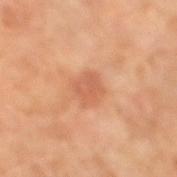biopsy status: imaged on a skin check; not biopsied
site: the right lower leg
patient: female, roughly 70 years of age
lighting: cross-polarized illumination
image: ~15 mm crop, total-body skin-cancer survey
size: about 3 mm